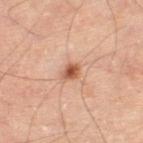The lesion was photographed on a routine skin check and not biopsied; there is no pathology result. From the leg. The total-body-photography lesion software estimated a lesion area of about 3.5 mm² and a shape eccentricity near 0.5. The analysis additionally found a border-irregularity index near 2.5/10 and a within-lesion color-variation index near 3.5/10. The analysis additionally found a nevus-likeness score of about 95/100 and a lesion-detection confidence of about 100/100. The patient is a male about 65 years old. A 15 mm crop from a total-body photograph taken for skin-cancer surveillance. Measured at roughly 2 mm in maximum diameter.